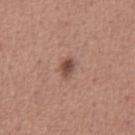Recorded during total-body skin imaging; not selected for excision or biopsy.
A lesion tile, about 15 mm wide, cut from a 3D total-body photograph.
The patient is a male aged around 45.
Located on the abdomen.
Automated tile analysis of the lesion measured a lesion–skin lightness drop of about 12 and a normalized lesion–skin contrast near 8.5. It also reported a classifier nevus-likeness of about 95/100 and lesion-presence confidence of about 100/100.
Approximately 2.5 mm at its widest.